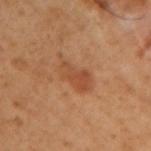Assessment:
Captured during whole-body skin photography for melanoma surveillance; the lesion was not biopsied.
Acquisition and patient details:
The tile uses cross-polarized illumination. The lesion is on the right upper arm. The patient is a male aged 48 to 52. The lesion-visualizer software estimated about 8 CIELAB-L* units darker than the surrounding skin and a normalized border contrast of about 6. The software also gave a border-irregularity index near 4.5/10, a color-variation rating of about 4/10, and radial color variation of about 1.5. And it measured a classifier nevus-likeness of about 25/100. The recorded lesion diameter is about 5 mm. A roughly 15 mm field-of-view crop from a total-body skin photograph.